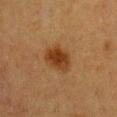Recorded during total-body skin imaging; not selected for excision or biopsy.
The tile uses cross-polarized illumination.
The lesion's longest dimension is about 3.5 mm.
A female patient, approximately 40 years of age.
Cropped from a total-body skin-imaging series; the visible field is about 15 mm.
On the front of the torso.